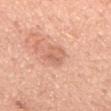Part of a total-body skin-imaging series; this lesion was reviewed on a skin check and was not flagged for biopsy. The subject is a male in their mid-60s. From the chest. A lesion tile, about 15 mm wide, cut from a 3D total-body photograph. The total-body-photography lesion software estimated a lesion color around L≈64 a*≈25 b*≈32 in CIELAB and a lesion–skin lightness drop of about 8. The software also gave a border-irregularity rating of about 3/10, a within-lesion color-variation index near 5/10, and radial color variation of about 1.5. Approximately 3 mm at its widest.